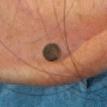biopsy status — total-body-photography surveillance lesion; no biopsy
imaging modality — total-body-photography crop, ~15 mm field of view
subject — female, aged approximately 50
anatomic site — the head or neck